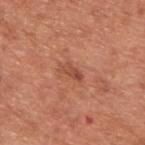This lesion was catalogued during total-body skin photography and was not selected for biopsy. Automated tile analysis of the lesion measured border irregularity of about 2.5 on a 0–10 scale and a peripheral color-asymmetry measure near 0. The software also gave a classifier nevus-likeness of about 5/100 and lesion-presence confidence of about 100/100. Approximately 2.5 mm at its widest. The lesion is located on the upper back. A 15 mm close-up tile from a total-body photography series done for melanoma screening. The subject is a male aged around 65.